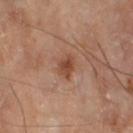Recorded during total-body skin imaging; not selected for excision or biopsy. Captured under cross-polarized illumination. Measured at roughly 3 mm in maximum diameter. From the leg. The total-body-photography lesion software estimated an area of roughly 4.5 mm², an outline eccentricity of about 0.75 (0 = round, 1 = elongated), and two-axis asymmetry of about 0.25. The analysis additionally found a lesion color around L≈46 a*≈23 b*≈31 in CIELAB and about 10 CIELAB-L* units darker than the surrounding skin. A 15 mm close-up extracted from a 3D total-body photography capture. A male patient, aged 63–67.